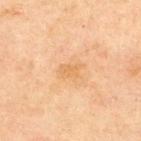Part of a total-body skin-imaging series; this lesion was reviewed on a skin check and was not flagged for biopsy.